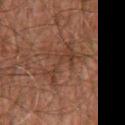This lesion was catalogued during total-body skin photography and was not selected for biopsy.
A male patient, aged around 60.
Located on the chest.
A 15 mm close-up tile from a total-body photography series done for melanoma screening.